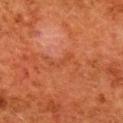Impression:
Captured during whole-body skin photography for melanoma surveillance; the lesion was not biopsied.
Image and clinical context:
The subject is a female roughly 50 years of age. A region of skin cropped from a whole-body photographic capture, roughly 15 mm wide. The lesion is on the upper back. The lesion's longest dimension is about 3.5 mm.